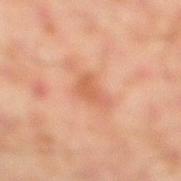{"image": {"source": "total-body photography crop", "field_of_view_mm": 15}, "lesion_size": {"long_diameter_mm_approx": 3.0}, "patient": {"sex": "male", "age_approx": 45}, "site": "right lower leg", "lighting": "cross-polarized"}